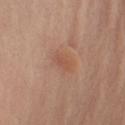illumination: white-light; lesion size: about 3 mm; patient: male, aged around 60; location: the right upper arm; imaging modality: total-body-photography crop, ~15 mm field of view.